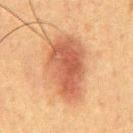{
  "biopsy_status": "not biopsied; imaged during a skin examination",
  "image": {
    "source": "total-body photography crop",
    "field_of_view_mm": 15
  },
  "patient": {
    "sex": "male",
    "age_approx": 50
  },
  "lesion_size": {
    "long_diameter_mm_approx": 8.0
  },
  "site": "chest",
  "lighting": "cross-polarized"
}